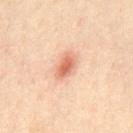notes = catalogued during a skin exam; not biopsied | imaging modality = 15 mm crop, total-body photography | patient = male, aged around 35 | location = the mid back | size = ≈3.5 mm | automated lesion analysis = a footprint of about 6.5 mm², a shape eccentricity near 0.8, and two-axis asymmetry of about 0.2 | illumination = cross-polarized.